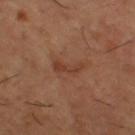Impression: The lesion was photographed on a routine skin check and not biopsied; there is no pathology result. Image and clinical context: On the right thigh. Automated image analysis of the tile measured internal color variation of about 0.5 on a 0–10 scale and radial color variation of about 0. Imaged with cross-polarized lighting. The patient is a male approximately 65 years of age. A roughly 15 mm field-of-view crop from a total-body skin photograph. About 3.5 mm across.